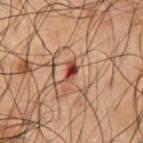notes: total-body-photography surveillance lesion; no biopsy
TBP lesion metrics: an average lesion color of about L≈33 a*≈23 b*≈22 (CIELAB), roughly 12 lightness units darker than nearby skin, and a lesion-to-skin contrast of about 11 (normalized; higher = more distinct); a classifier nevus-likeness of about 0/100 and lesion-presence confidence of about 100/100
site: the abdomen
patient: male, in their 50s
diameter: about 2.5 mm
tile lighting: cross-polarized
imaging modality: 15 mm crop, total-body photography